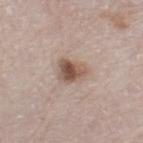{
  "biopsy_status": "not biopsied; imaged during a skin examination",
  "image": {
    "source": "total-body photography crop",
    "field_of_view_mm": 15
  },
  "lighting": "white-light",
  "lesion_size": {
    "long_diameter_mm_approx": 3.5
  },
  "patient": {
    "sex": "male",
    "age_approx": 80
  },
  "site": "right lower leg"
}